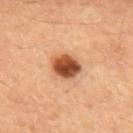Assessment:
Imaged during a routine full-body skin examination; the lesion was not biopsied and no histopathology is available.
Clinical summary:
A male subject aged 58–62. The recorded lesion diameter is about 3.5 mm. This image is a 15 mm lesion crop taken from a total-body photograph. Automated image analysis of the tile measured a footprint of about 9 mm², an outline eccentricity of about 0.55 (0 = round, 1 = elongated), and two-axis asymmetry of about 0.15. It also reported a mean CIELAB color near L≈44 a*≈24 b*≈33, roughly 17 lightness units darker than nearby skin, and a normalized lesion–skin contrast near 12. The software also gave a classifier nevus-likeness of about 100/100 and a lesion-detection confidence of about 100/100. This is a cross-polarized tile.